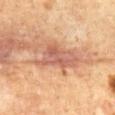Assessment: This lesion was catalogued during total-body skin photography and was not selected for biopsy. Image and clinical context: From the abdomen. About 6 mm across. This is a cross-polarized tile. Automated image analysis of the tile measured an area of roughly 11 mm² and an outline eccentricity of about 0.85 (0 = round, 1 = elongated). And it measured an automated nevus-likeness rating near 0 out of 100 and a detector confidence of about 100 out of 100 that the crop contains a lesion. A male patient, aged 63–67. A lesion tile, about 15 mm wide, cut from a 3D total-body photograph.